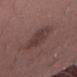Impression: The lesion was photographed on a routine skin check and not biopsied; there is no pathology result. Acquisition and patient details: Imaged with white-light lighting. A male patient, aged approximately 50. The total-body-photography lesion software estimated a lesion color around L≈38 a*≈18 b*≈19 in CIELAB. The lesion is located on the right thigh. The recorded lesion diameter is about 5.5 mm. Cropped from a whole-body photographic skin survey; the tile spans about 15 mm.